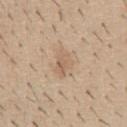follow-up = imaged on a skin check; not biopsied
body site = the abdomen
subject = male, approximately 40 years of age
lighting = white-light
lesion diameter = ~3.5 mm (longest diameter)
image source = ~15 mm crop, total-body skin-cancer survey